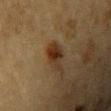• image-analysis metrics: a border-irregularity index near 4/10, internal color variation of about 3.5 on a 0–10 scale, and peripheral color asymmetry of about 1
• anatomic site: the left upper arm
• image: 15 mm crop, total-body photography
• subject: male, aged approximately 85 or older
• lighting: cross-polarized
• diameter: ≈4 mm